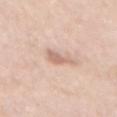The lesion was photographed on a routine skin check and not biopsied; there is no pathology result.